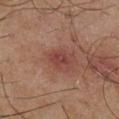<tbp_lesion>
<biopsy_status>not biopsied; imaged during a skin examination</biopsy_status>
<patient>
  <sex>male</sex>
  <age_approx>65</age_approx>
</patient>
<lighting>cross-polarized</lighting>
<image>
  <source>total-body photography crop</source>
  <field_of_view_mm>15</field_of_view_mm>
</image>
<site>right lower leg</site>
</tbp_lesion>